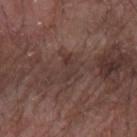Imaged during a routine full-body skin examination; the lesion was not biopsied and no histopathology is available.